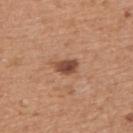Part of a total-body skin-imaging series; this lesion was reviewed on a skin check and was not flagged for biopsy.
A male subject, aged approximately 55.
The tile uses white-light illumination.
The lesion is on the right upper arm.
Measured at roughly 3 mm in maximum diameter.
Cropped from a whole-body photographic skin survey; the tile spans about 15 mm.